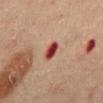The lesion was tiled from a total-body skin photograph and was not biopsied. The total-body-photography lesion software estimated a footprint of about 4 mm², an outline eccentricity of about 0.75 (0 = round, 1 = elongated), and a shape-asymmetry score of about 0.25 (0 = symmetric). The software also gave an average lesion color of about L≈38 a*≈30 b*≈25 (CIELAB), about 15 CIELAB-L* units darker than the surrounding skin, and a lesion-to-skin contrast of about 12.5 (normalized; higher = more distinct). A male subject roughly 45 years of age. The lesion's longest dimension is about 3 mm. Cropped from a whole-body photographic skin survey; the tile spans about 15 mm. Captured under cross-polarized illumination. On the abdomen.